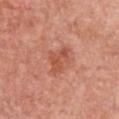notes: total-body-photography surveillance lesion; no biopsy
size: ~3.5 mm (longest diameter)
tile lighting: white-light
acquisition: ~15 mm tile from a whole-body skin photo
site: the chest
subject: female, aged approximately 60